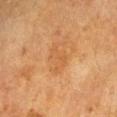Part of a total-body skin-imaging series; this lesion was reviewed on a skin check and was not flagged for biopsy. The lesion is on the right forearm. The tile uses cross-polarized illumination. Longest diameter approximately 3.5 mm. A female subject aged 53 to 57. An algorithmic analysis of the crop reported a footprint of about 5 mm², an outline eccentricity of about 0.75 (0 = round, 1 = elongated), and a shape-asymmetry score of about 0.55 (0 = symmetric). The analysis additionally found a mean CIELAB color near L≈47 a*≈20 b*≈36 and a normalized lesion–skin contrast near 4.5. A lesion tile, about 15 mm wide, cut from a 3D total-body photograph.